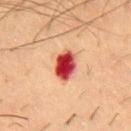follow-up = imaged on a skin check; not biopsied | acquisition = ~15 mm crop, total-body skin-cancer survey | tile lighting = cross-polarized illumination | patient = male, approximately 55 years of age | lesion diameter = ≈3.5 mm | location = the upper back.